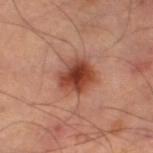The lesion was tiled from a total-body skin photograph and was not biopsied. The lesion's longest dimension is about 4.5 mm. Cropped from a total-body skin-imaging series; the visible field is about 15 mm. The tile uses cross-polarized illumination. From the left thigh.